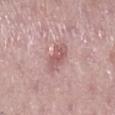The lesion was photographed on a routine skin check and not biopsied; there is no pathology result. From the right lower leg. Cropped from a total-body skin-imaging series; the visible field is about 15 mm. A female subject about 40 years old. The recorded lesion diameter is about 4 mm. The tile uses white-light illumination.